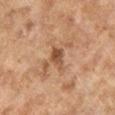follow-up: total-body-photography surveillance lesion; no biopsy | patient: female, aged 58–62 | tile lighting: cross-polarized illumination | site: the left lower leg | image: 15 mm crop, total-body photography.